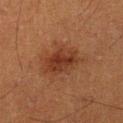Q: Is there a histopathology result?
A: imaged on a skin check; not biopsied
Q: Where on the body is the lesion?
A: the leg
Q: Illumination type?
A: cross-polarized illumination
Q: Lesion size?
A: ~4.5 mm (longest diameter)
Q: Who is the patient?
A: male, about 40 years old
Q: What is the imaging modality?
A: ~15 mm crop, total-body skin-cancer survey
Q: What did automated image analysis measure?
A: a border-irregularity rating of about 2.5/10, internal color variation of about 4 on a 0–10 scale, and a peripheral color-asymmetry measure near 1; an automated nevus-likeness rating near 60 out of 100